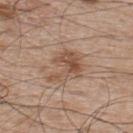{"biopsy_status": "not biopsied; imaged during a skin examination", "site": "back", "image": {"source": "total-body photography crop", "field_of_view_mm": 15}, "patient": {"sex": "male", "age_approx": 50}, "automated_metrics": {"eccentricity": 0.65, "shape_asymmetry": 0.4, "cielab_L": 52, "cielab_a": 18, "cielab_b": 29, "vs_skin_darker_L": 9.0, "vs_skin_contrast_norm": 7.0, "border_irregularity_0_10": 5.5, "color_variation_0_10": 5.0, "peripheral_color_asymmetry": 1.5}, "lesion_size": {"long_diameter_mm_approx": 4.5}, "lighting": "white-light"}